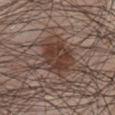Context:
Automated tile analysis of the lesion measured an area of roughly 13 mm², a shape eccentricity near 0.6, and a symmetry-axis asymmetry near 0.2. It also reported a border-irregularity rating of about 2.5/10 and peripheral color asymmetry of about 1.5. It also reported an automated nevus-likeness rating near 95 out of 100 and a lesion-detection confidence of about 100/100. A close-up tile cropped from a whole-body skin photograph, about 15 mm across. Located on the chest. A male subject in their mid- to late 60s.